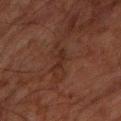The lesion was photographed on a routine skin check and not biopsied; there is no pathology result.
A region of skin cropped from a whole-body photographic capture, roughly 15 mm wide.
From the arm.
A male patient, in their mid- to late 60s.